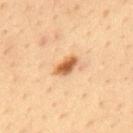Assessment:
Imaged during a routine full-body skin examination; the lesion was not biopsied and no histopathology is available.
Background:
Approximately 3 mm at its widest. The lesion is located on the mid back. A region of skin cropped from a whole-body photographic capture, roughly 15 mm wide. This is a cross-polarized tile. A male patient about 35 years old.